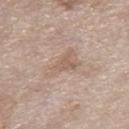The lesion was tiled from a total-body skin photograph and was not biopsied. This image is a 15 mm lesion crop taken from a total-body photograph. A male patient, roughly 60 years of age. About 4.5 mm across. Captured under white-light illumination. The lesion is located on the right thigh.